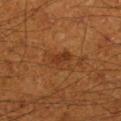• follow-up · total-body-photography surveillance lesion; no biopsy
• patient · male, aged 58–62
• body site · the right lower leg
• lesion diameter · ~3 mm (longest diameter)
• image · ~15 mm tile from a whole-body skin photo